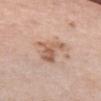<lesion>
<automated_metrics>
  <border_irregularity_0_10>6.0</border_irregularity_0_10>
  <color_variation_0_10>4.5</color_variation_0_10>
  <nevus_likeness_0_100>10</nevus_likeness_0_100>
  <lesion_detection_confidence_0_100>100</lesion_detection_confidence_0_100>
</automated_metrics>
<image>
  <source>total-body photography crop</source>
  <field_of_view_mm>15</field_of_view_mm>
</image>
<patient>
  <sex>female</sex>
  <age_approx>40</age_approx>
</patient>
<site>chest</site>
</lesion>